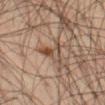subject — male, about 45 years old | size — ≈3.5 mm | image source — ~15 mm crop, total-body skin-cancer survey | tile lighting — cross-polarized illumination | anatomic site — the left thigh | image-analysis metrics — an area of roughly 5.5 mm², an eccentricity of roughly 0.8, and a symmetry-axis asymmetry near 0.55; a border-irregularity index near 6/10 and radial color variation of about 4; a classifier nevus-likeness of about 25/100 and a detector confidence of about 100 out of 100 that the crop contains a lesion.